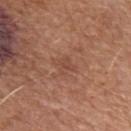• notes · imaged on a skin check; not biopsied
• image · total-body-photography crop, ~15 mm field of view
• automated lesion analysis · a nevus-likeness score of about 0/100
• subject · male, aged approximately 65
• location · the arm
• lighting · white-light